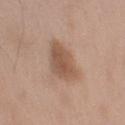Clinical impression:
No biopsy was performed on this lesion — it was imaged during a full skin examination and was not determined to be concerning.
Clinical summary:
The lesion is located on the right upper arm. A 15 mm close-up extracted from a 3D total-body photography capture. Imaged with white-light lighting. The patient is a male aged around 50.